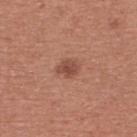Assessment:
No biopsy was performed on this lesion — it was imaged during a full skin examination and was not determined to be concerning.
Context:
The lesion's longest dimension is about 2.5 mm. The lesion is on the upper back. The tile uses white-light illumination. A female subject roughly 50 years of age. A lesion tile, about 15 mm wide, cut from a 3D total-body photograph.